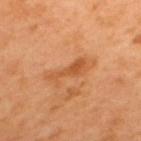Q: Is there a histopathology result?
A: imaged on a skin check; not biopsied
Q: What is the anatomic site?
A: the upper back
Q: How large is the lesion?
A: ~4.5 mm (longest diameter)
Q: What lighting was used for the tile?
A: cross-polarized illumination
Q: Patient demographics?
A: male, roughly 50 years of age
Q: How was this image acquired?
A: ~15 mm crop, total-body skin-cancer survey